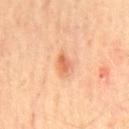image:
  source: total-body photography crop
  field_of_view_mm: 15
patient:
  sex: male
  age_approx: 65
lesion_size:
  long_diameter_mm_approx: 2.5
lighting: cross-polarized
site: chest
automated_metrics:
  area_mm2_approx: 3.5
  eccentricity: 0.7
  shape_asymmetry: 0.3
  cielab_L: 66
  cielab_a: 28
  cielab_b: 39
  vs_skin_contrast_norm: 7.0
  border_irregularity_0_10: 3.0
  color_variation_0_10: 3.0